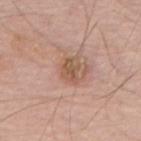Q: What lighting was used for the tile?
A: white-light
Q: What is the lesion's diameter?
A: ≈3 mm
Q: Patient demographics?
A: male, in their 70s
Q: Automated lesion metrics?
A: a lesion area of about 5 mm² and a shape-asymmetry score of about 0.25 (0 = symmetric); an average lesion color of about L≈55 a*≈19 b*≈31 (CIELAB), a lesion–skin lightness drop of about 9, and a lesion-to-skin contrast of about 7 (normalized; higher = more distinct)
Q: Where on the body is the lesion?
A: the mid back
Q: How was this image acquired?
A: ~15 mm crop, total-body skin-cancer survey